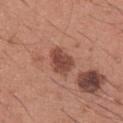Captured during whole-body skin photography for melanoma surveillance; the lesion was not biopsied. The lesion-visualizer software estimated an average lesion color of about L≈46 a*≈24 b*≈27 (CIELAB), a lesion–skin lightness drop of about 12, and a normalized border contrast of about 9. The analysis additionally found a within-lesion color-variation index near 3/10 and a peripheral color-asymmetry measure near 1. The analysis additionally found a nevus-likeness score of about 5/100 and lesion-presence confidence of about 100/100. This is a white-light tile. Cropped from a total-body skin-imaging series; the visible field is about 15 mm. On the arm. Measured at roughly 3.5 mm in maximum diameter. A male patient aged around 35.